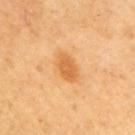Assessment: Recorded during total-body skin imaging; not selected for excision or biopsy. Image and clinical context: The lesion-visualizer software estimated an area of roughly 6.5 mm², an outline eccentricity of about 0.8 (0 = round, 1 = elongated), and a shape-asymmetry score of about 0.25 (0 = symmetric). And it measured an average lesion color of about L≈64 a*≈25 b*≈47 (CIELAB), a lesion–skin lightness drop of about 10, and a normalized lesion–skin contrast near 7. The analysis additionally found border irregularity of about 2 on a 0–10 scale and a color-variation rating of about 2/10. From the left arm. A close-up tile cropped from a whole-body skin photograph, about 15 mm across. Approximately 3.5 mm at its widest. The subject is a female aged 43–47.